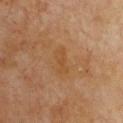Findings:
• body site: the chest
• subject: female, in their 60s
• lighting: cross-polarized
• diameter: about 3.5 mm
• imaging modality: total-body-photography crop, ~15 mm field of view